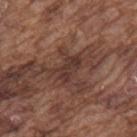| feature | finding |
|---|---|
| follow-up | imaged on a skin check; not biopsied |
| lesion diameter | ≈4 mm |
| lighting | white-light |
| anatomic site | the upper back |
| patient | male, approximately 75 years of age |
| TBP lesion metrics | an automated nevus-likeness rating near 0 out of 100 and lesion-presence confidence of about 75/100 |
| image source | total-body-photography crop, ~15 mm field of view |